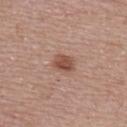Notes:
* workup: total-body-photography surveillance lesion; no biopsy
* imaging modality: 15 mm crop, total-body photography
* subject: female, approximately 65 years of age
* body site: the upper back